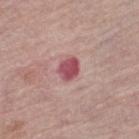| key | value |
|---|---|
| biopsy status | imaged on a skin check; not biopsied |
| anatomic site | the left thigh |
| image source | 15 mm crop, total-body photography |
| subject | male, in their mid-70s |